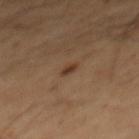| feature | finding |
|---|---|
| follow-up | imaged on a skin check; not biopsied |
| anatomic site | the mid back |
| subject | male, aged 48–52 |
| acquisition | ~15 mm tile from a whole-body skin photo |
| diameter | about 2 mm |
| automated lesion analysis | an area of roughly 2 mm² and an outline eccentricity of about 0.8 (0 = round, 1 = elongated); a border-irregularity index near 2.5/10, a within-lesion color-variation index near 1/10, and a peripheral color-asymmetry measure near 0.5 |
| illumination | cross-polarized |